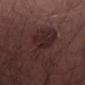Assessment:
The lesion was tiled from a total-body skin photograph and was not biopsied.
Context:
Automated image analysis of the tile measured an area of roughly 14 mm², a shape eccentricity near 0.95, and a symmetry-axis asymmetry near 0.5. The analysis additionally found a lesion–skin lightness drop of about 6 and a lesion-to-skin contrast of about 6.5 (normalized; higher = more distinct). And it measured internal color variation of about 2.5 on a 0–10 scale and radial color variation of about 1. The analysis additionally found a classifier nevus-likeness of about 10/100 and a lesion-detection confidence of about 90/100. The recorded lesion diameter is about 8 mm. On the lower back. A close-up tile cropped from a whole-body skin photograph, about 15 mm across. A male patient, in their 50s.